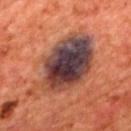* workup: catalogued during a skin exam; not biopsied
* image source: 15 mm crop, total-body photography
* image-analysis metrics: a lesion area of about 35 mm², a shape eccentricity near 0.8, and a shape-asymmetry score of about 0.25 (0 = symmetric); a border-irregularity rating of about 4/10, a color-variation rating of about 10/10, and peripheral color asymmetry of about 4.5
* size: about 10 mm
* subject: male, approximately 65 years of age
* lighting: cross-polarized
* anatomic site: the back